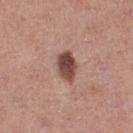Background: About 3.5 mm across. Located on the left thigh. Imaged with white-light lighting. The subject is a female in their 40s. A 15 mm crop from a total-body photograph taken for skin-cancer surveillance.